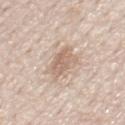Context:
A 15 mm close-up tile from a total-body photography series done for melanoma screening. Approximately 5 mm at its widest. A male subject about 50 years old. The lesion-visualizer software estimated a footprint of about 9.5 mm² and two-axis asymmetry of about 0.3. Captured under white-light illumination. On the front of the torso.